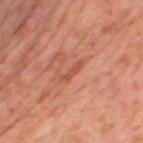notes = imaged on a skin check; not biopsied
image = ~15 mm crop, total-body skin-cancer survey
subject = female, roughly 50 years of age
illumination = cross-polarized illumination
image-analysis metrics = a footprint of about 3 mm², a shape eccentricity near 0.95, and two-axis asymmetry of about 0.35; a mean CIELAB color near L≈51 a*≈28 b*≈34 and a normalized lesion–skin contrast near 6; a border-irregularity rating of about 4.5/10, a color-variation rating of about 0/10, and peripheral color asymmetry of about 0
location = the left thigh
diameter = about 3 mm